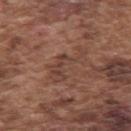{
  "biopsy_status": "not biopsied; imaged during a skin examination"
}